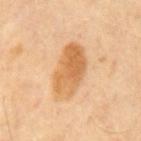Q: Is there a histopathology result?
A: catalogued during a skin exam; not biopsied
Q: How was this image acquired?
A: total-body-photography crop, ~15 mm field of view
Q: Patient demographics?
A: male, aged approximately 70
Q: How large is the lesion?
A: ≈6 mm
Q: Illumination type?
A: cross-polarized illumination
Q: What is the anatomic site?
A: the mid back